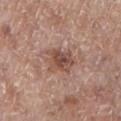| key | value |
|---|---|
| follow-up | catalogued during a skin exam; not biopsied |
| subject | female, in their mid-70s |
| illumination | white-light illumination |
| site | the left lower leg |
| acquisition | ~15 mm tile from a whole-body skin photo |